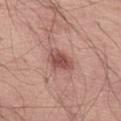Located on the abdomen. Approximately 3.5 mm at its widest. The patient is a male in their mid-50s. A 15 mm close-up tile from a total-body photography series done for melanoma screening. Imaged with white-light lighting.